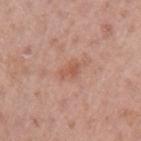Impression: Captured during whole-body skin photography for melanoma surveillance; the lesion was not biopsied. Clinical summary: A male patient aged around 40. A close-up tile cropped from a whole-body skin photograph, about 15 mm across. The lesion is on the left upper arm. Automated image analysis of the tile measured a footprint of about 2.5 mm², an outline eccentricity of about 0.85 (0 = round, 1 = elongated), and two-axis asymmetry of about 0.35. And it measured an average lesion color of about L≈55 a*≈24 b*≈30 (CIELAB) and about 8 CIELAB-L* units darker than the surrounding skin. The software also gave an automated nevus-likeness rating near 0 out of 100. Longest diameter approximately 2.5 mm.